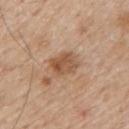Q: Is there a histopathology result?
A: total-body-photography surveillance lesion; no biopsy
Q: What is the lesion's diameter?
A: ≈3.5 mm
Q: What lighting was used for the tile?
A: white-light illumination
Q: What kind of image is this?
A: ~15 mm crop, total-body skin-cancer survey
Q: Patient demographics?
A: male, aged 58–62
Q: What is the anatomic site?
A: the mid back